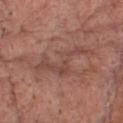This lesion was catalogued during total-body skin photography and was not selected for biopsy.
Approximately 8 mm at its widest.
A lesion tile, about 15 mm wide, cut from a 3D total-body photograph.
The lesion is on the chest.
A male subject, aged approximately 60.
Imaged with white-light lighting.